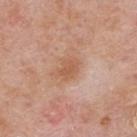The lesion was tiled from a total-body skin photograph and was not biopsied. The patient is a male roughly 75 years of age. This is a white-light tile. Approximately 3 mm at its widest. From the back. A 15 mm close-up extracted from a 3D total-body photography capture. Automated image analysis of the tile measured a footprint of about 5 mm², an eccentricity of roughly 0.7, and two-axis asymmetry of about 0.25. The analysis additionally found an automated nevus-likeness rating near 5 out of 100 and a lesion-detection confidence of about 100/100.